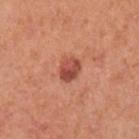<lesion>
<biopsy_status>not biopsied; imaged during a skin examination</biopsy_status>
<lighting>white-light</lighting>
<site>left upper arm</site>
<automated_metrics>
  <cielab_L>50</cielab_L>
  <cielab_a>31</cielab_a>
  <cielab_b>31</cielab_b>
  <vs_skin_darker_L>13.0</vs_skin_darker_L>
  <vs_skin_contrast_norm>8.5</vs_skin_contrast_norm>
  <lesion_detection_confidence_0_100>100</lesion_detection_confidence_0_100>
</automated_metrics>
<image>
  <source>total-body photography crop</source>
  <field_of_view_mm>15</field_of_view_mm>
</image>
<lesion_size>
  <long_diameter_mm_approx>3.0</long_diameter_mm_approx>
</lesion_size>
<patient>
  <sex>female</sex>
  <age_approx>40</age_approx>
</patient>
</lesion>